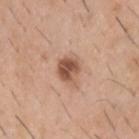Q: What are the patient's age and sex?
A: male, roughly 40 years of age
Q: How was this image acquired?
A: 15 mm crop, total-body photography
Q: Where on the body is the lesion?
A: the upper back